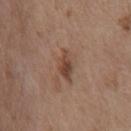{
  "biopsy_status": "not biopsied; imaged during a skin examination",
  "automated_metrics": {
    "area_mm2_approx": 5.0,
    "eccentricity": 0.85,
    "shape_asymmetry": 0.3,
    "cielab_L": 44,
    "cielab_a": 19,
    "cielab_b": 27,
    "vs_skin_darker_L": 9.0,
    "nevus_likeness_0_100": 65,
    "lesion_detection_confidence_0_100": 100
  },
  "site": "chest",
  "patient": {
    "sex": "female",
    "age_approx": 65
  },
  "image": {
    "source": "total-body photography crop",
    "field_of_view_mm": 15
  }
}